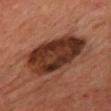– biopsy status — catalogued during a skin exam; not biopsied
– location — the back
– subject — male, approximately 60 years of age
– tile lighting — cross-polarized illumination
– image — ~15 mm crop, total-body skin-cancer survey